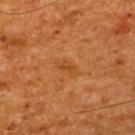Q: Was this lesion biopsied?
A: catalogued during a skin exam; not biopsied
Q: What is the imaging modality?
A: ~15 mm crop, total-body skin-cancer survey
Q: Where on the body is the lesion?
A: the upper back
Q: Patient demographics?
A: male, aged approximately 65